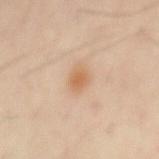Assessment: Captured during whole-body skin photography for melanoma surveillance; the lesion was not biopsied. Background: A male subject aged 53 to 57. The lesion is located on the chest. Cropped from a total-body skin-imaging series; the visible field is about 15 mm. Automated image analysis of the tile measured border irregularity of about 1.5 on a 0–10 scale, internal color variation of about 2 on a 0–10 scale, and peripheral color asymmetry of about 0.5.